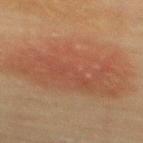Part of a total-body skin-imaging series; this lesion was reviewed on a skin check and was not flagged for biopsy.
An algorithmic analysis of the crop reported a lesion color around L≈43 a*≈20 b*≈28 in CIELAB, about 6 CIELAB-L* units darker than the surrounding skin, and a normalized border contrast of about 5.5.
Located on the back.
Cropped from a total-body skin-imaging series; the visible field is about 15 mm.
A female patient aged approximately 65.